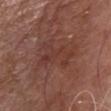Assessment: Part of a total-body skin-imaging series; this lesion was reviewed on a skin check and was not flagged for biopsy. Image and clinical context: Imaged with white-light lighting. Located on the chest. The subject is a male approximately 80 years of age. This image is a 15 mm lesion crop taken from a total-body photograph. Longest diameter approximately 5.5 mm.